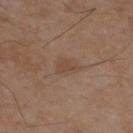The lesion was tiled from a total-body skin photograph and was not biopsied. A region of skin cropped from a whole-body photographic capture, roughly 15 mm wide. On the upper back. Captured under cross-polarized illumination. A male subject, in their mid-50s.